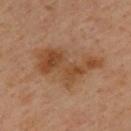<lesion>
  <patient>
    <sex>male</sex>
    <age_approx>40</age_approx>
  </patient>
  <site>upper back</site>
  <image>
    <source>total-body photography crop</source>
    <field_of_view_mm>15</field_of_view_mm>
  </image>
</lesion>